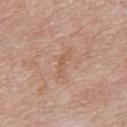- notes — total-body-photography surveillance lesion; no biopsy
- image source — ~15 mm crop, total-body skin-cancer survey
- subject — male, in their mid-80s or older
- anatomic site — the upper back